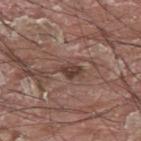{
  "biopsy_status": "not biopsied; imaged during a skin examination",
  "lighting": "white-light",
  "automated_metrics": {
    "nevus_likeness_0_100": 0,
    "lesion_detection_confidence_0_100": 95
  },
  "image": {
    "source": "total-body photography crop",
    "field_of_view_mm": 15
  },
  "patient": {
    "sex": "male",
    "age_approx": 40
  },
  "site": "upper back"
}